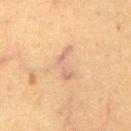Assessment: This lesion was catalogued during total-body skin photography and was not selected for biopsy. Image and clinical context: A male patient approximately 50 years of age. The lesion is on the chest. Automated tile analysis of the lesion measured a lesion color around L≈53 a*≈15 b*≈26 in CIELAB, about 6 CIELAB-L* units darker than the surrounding skin, and a normalized lesion–skin contrast near 5.5. The software also gave a detector confidence of about 85 out of 100 that the crop contains a lesion. Cropped from a total-body skin-imaging series; the visible field is about 15 mm. Imaged with cross-polarized lighting.